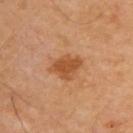– workup: imaged on a skin check; not biopsied
– imaging modality: ~15 mm crop, total-body skin-cancer survey
– subject: male, aged 68–72
– lesion diameter: ~3.5 mm (longest diameter)
– TBP lesion metrics: an area of roughly 7.5 mm², a shape eccentricity near 0.65, and a shape-asymmetry score of about 0.3 (0 = symmetric); an average lesion color of about L≈49 a*≈25 b*≈38 (CIELAB), about 10 CIELAB-L* units darker than the surrounding skin, and a lesion-to-skin contrast of about 8 (normalized; higher = more distinct); a border-irregularity rating of about 3/10, a color-variation rating of about 2/10, and peripheral color asymmetry of about 0.5
– anatomic site: the upper back
– lighting: cross-polarized